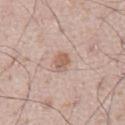Notes:
• workup · catalogued during a skin exam; not biopsied
• tile lighting · white-light
• image source · 15 mm crop, total-body photography
• anatomic site · the front of the torso
• subject · male, in their mid- to late 60s
• size · ~2.5 mm (longest diameter)